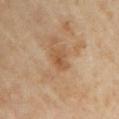Imaged during a routine full-body skin examination; the lesion was not biopsied and no histopathology is available. Longest diameter approximately 3 mm. This image is a 15 mm lesion crop taken from a total-body photograph. A female subject, aged approximately 60. An algorithmic analysis of the crop reported roughly 8 lightness units darker than nearby skin and a lesion-to-skin contrast of about 6 (normalized; higher = more distinct). The analysis additionally found a border-irregularity index near 3/10, a within-lesion color-variation index near 3.5/10, and peripheral color asymmetry of about 1. The software also gave a nevus-likeness score of about 0/100 and lesion-presence confidence of about 100/100. Imaged with cross-polarized lighting. Located on the left upper arm.